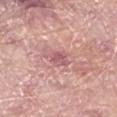The lesion was tiled from a total-body skin photograph and was not biopsied. From the leg. A female patient, aged 58 to 62. Cropped from a whole-body photographic skin survey; the tile spans about 15 mm.